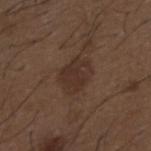No biopsy was performed on this lesion — it was imaged during a full skin examination and was not determined to be concerning. Cropped from a whole-body photographic skin survey; the tile spans about 15 mm. Imaged with white-light lighting. The recorded lesion diameter is about 4 mm. The total-body-photography lesion software estimated a border-irregularity index near 2/10, internal color variation of about 2.5 on a 0–10 scale, and a peripheral color-asymmetry measure near 1. The software also gave an automated nevus-likeness rating near 10 out of 100 and lesion-presence confidence of about 100/100. The lesion is located on the mid back. The subject is a male in their 50s.